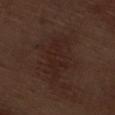Q: Was this lesion biopsied?
A: imaged on a skin check; not biopsied
Q: Lesion size?
A: ~6 mm (longest diameter)
Q: What kind of image is this?
A: 15 mm crop, total-body photography
Q: How was the tile lit?
A: white-light illumination
Q: Lesion location?
A: the leg
Q: What are the patient's age and sex?
A: male, in their 70s